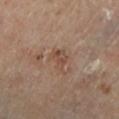Part of a total-body skin-imaging series; this lesion was reviewed on a skin check and was not flagged for biopsy. A 15 mm close-up extracted from a 3D total-body photography capture. On the left lower leg. Captured under cross-polarized illumination. A female subject about 60 years old. The recorded lesion diameter is about 3 mm.